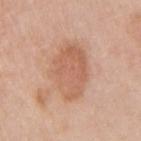workup: total-body-photography surveillance lesion; no biopsy
tile lighting: white-light
image: 15 mm crop, total-body photography
location: the right upper arm
patient: female, aged approximately 40
automated lesion analysis: a lesion color around L≈61 a*≈22 b*≈32 in CIELAB, about 9 CIELAB-L* units darker than the surrounding skin, and a lesion-to-skin contrast of about 6 (normalized; higher = more distinct)
diameter: ~6.5 mm (longest diameter)